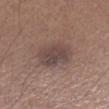The lesion was photographed on a routine skin check and not biopsied; there is no pathology result.
A male subject, roughly 65 years of age.
Automated image analysis of the tile measured an area of roughly 12 mm², a shape eccentricity near 0.8, and two-axis asymmetry of about 0.15.
A close-up tile cropped from a whole-body skin photograph, about 15 mm across.
Located on the left lower leg.